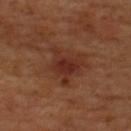The lesion was photographed on a routine skin check and not biopsied; there is no pathology result.
From the upper back.
The lesion-visualizer software estimated an area of roughly 10 mm², an outline eccentricity of about 0.5 (0 = round, 1 = elongated), and a shape-asymmetry score of about 0.45 (0 = symmetric).
A male patient aged approximately 70.
Cropped from a whole-body photographic skin survey; the tile spans about 15 mm.
The recorded lesion diameter is about 4.5 mm.
Captured under cross-polarized illumination.